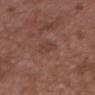workup: no biopsy performed (imaged during a skin exam)
patient: male, aged 48 to 52
image source: total-body-photography crop, ~15 mm field of view
site: the head or neck